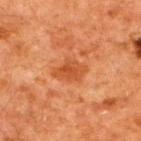  biopsy_status: not biopsied; imaged during a skin examination
  lesion_size:
    long_diameter_mm_approx: 4.0
  lighting: cross-polarized
  patient:
    sex: male
    age_approx: 60
  image:
    source: total-body photography crop
    field_of_view_mm: 15
  site: upper back